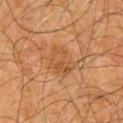biopsy_status: not biopsied; imaged during a skin examination
lesion_size:
  long_diameter_mm_approx: 3.5
lighting: cross-polarized
patient:
  sex: male
  age_approx: 60
image:
  source: total-body photography crop
  field_of_view_mm: 15
site: chest